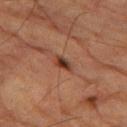Findings:
- workup · total-body-photography surveillance lesion; no biopsy
- patient · male, approximately 85 years of age or older
- diameter · about 2.5 mm
- automated lesion analysis · a lesion–skin lightness drop of about 11 and a normalized lesion–skin contrast near 10; a border-irregularity index near 3/10, a color-variation rating of about 5.5/10, and peripheral color asymmetry of about 1.5; an automated nevus-likeness rating near 85 out of 100 and lesion-presence confidence of about 100/100
- image · ~15 mm tile from a whole-body skin photo
- location · the left thigh